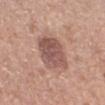Captured during whole-body skin photography for melanoma surveillance; the lesion was not biopsied.
A lesion tile, about 15 mm wide, cut from a 3D total-body photograph.
The recorded lesion diameter is about 4.5 mm.
From the right forearm.
A female patient, roughly 40 years of age.